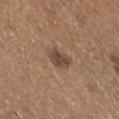- workup — catalogued during a skin exam; not biopsied
- imaging modality — total-body-photography crop, ~15 mm field of view
- location — the abdomen
- patient — male, aged approximately 65
- lighting — white-light illumination
- lesion size — about 3 mm